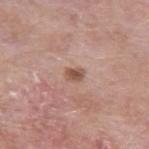| key | value |
|---|---|
| follow-up | imaged on a skin check; not biopsied |
| acquisition | ~15 mm crop, total-body skin-cancer survey |
| location | the left upper arm |
| subject | male, in their mid- to late 60s |